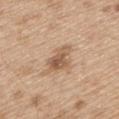– notes: no biopsy performed (imaged during a skin exam)
– TBP lesion metrics: a lesion area of about 6 mm², an eccentricity of roughly 0.9, and a symmetry-axis asymmetry near 0.35; a mean CIELAB color near L≈56 a*≈18 b*≈32, about 12 CIELAB-L* units darker than the surrounding skin, and a normalized lesion–skin contrast near 7.5; a border-irregularity rating of about 4/10 and a color-variation rating of about 3.5/10; an automated nevus-likeness rating near 15 out of 100
– imaging modality: ~15 mm tile from a whole-body skin photo
– patient: male, aged 68 to 72
– location: the mid back
– lighting: white-light
– size: about 4.5 mm